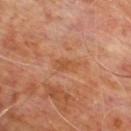The lesion was tiled from a total-body skin photograph and was not biopsied. The lesion is located on the upper back. A 15 mm crop from a total-body photograph taken for skin-cancer surveillance. Measured at roughly 2.5 mm in maximum diameter. A male patient, aged approximately 60. The tile uses cross-polarized illumination. The lesion-visualizer software estimated a mean CIELAB color near L≈49 a*≈24 b*≈36, roughly 6 lightness units darker than nearby skin, and a normalized border contrast of about 5.5. It also reported a lesion-detection confidence of about 100/100.